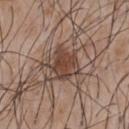Captured during whole-body skin photography for melanoma surveillance; the lesion was not biopsied. A close-up tile cropped from a whole-body skin photograph, about 15 mm across. The tile uses white-light illumination. Approximately 4 mm at its widest. The subject is a male aged around 50. On the chest.